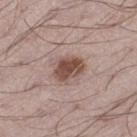The lesion was tiled from a total-body skin photograph and was not biopsied. A male patient, aged around 50. The lesion is on the leg. This is a white-light tile. This image is a 15 mm lesion crop taken from a total-body photograph. The total-body-photography lesion software estimated a footprint of about 7.5 mm² and two-axis asymmetry of about 0.2. The software also gave a mean CIELAB color near L≈48 a*≈19 b*≈24 and a normalized lesion–skin contrast near 10.5. The software also gave a within-lesion color-variation index near 3.5/10 and radial color variation of about 1. Approximately 3.5 mm at its widest.